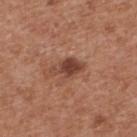Clinical impression: Imaged during a routine full-body skin examination; the lesion was not biopsied and no histopathology is available. Background: The recorded lesion diameter is about 4 mm. Imaged with white-light lighting. A 15 mm close-up tile from a total-body photography series done for melanoma screening. The lesion-visualizer software estimated a footprint of about 6 mm² and an eccentricity of roughly 0.85. The software also gave a border-irregularity rating of about 4/10, a color-variation rating of about 1.5/10, and a peripheral color-asymmetry measure near 0.5. And it measured an automated nevus-likeness rating near 65 out of 100 and a lesion-detection confidence of about 100/100. The patient is a male aged 63 to 67. From the upper back.